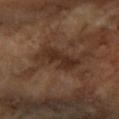The lesion was tiled from a total-body skin photograph and was not biopsied. Longest diameter approximately 5 mm. The subject is a female aged around 70. The lesion is located on the right forearm. A 15 mm close-up tile from a total-body photography series done for melanoma screening. This is a cross-polarized tile.